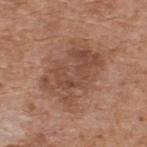Imaged during a routine full-body skin examination; the lesion was not biopsied and no histopathology is available. Longest diameter approximately 6.5 mm. Automated image analysis of the tile measured a within-lesion color-variation index near 4.5/10 and radial color variation of about 1.5. A male subject in their 60s. Cropped from a whole-body photographic skin survey; the tile spans about 15 mm. The lesion is located on the upper back. This is a white-light tile.